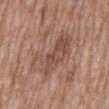notes = no biopsy performed (imaged during a skin exam) | patient = male, about 60 years old | imaging modality = 15 mm crop, total-body photography | tile lighting = white-light illumination | location = the abdomen.